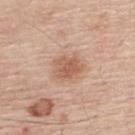Clinical impression: Imaged during a routine full-body skin examination; the lesion was not biopsied and no histopathology is available. Image and clinical context: The lesion-visualizer software estimated an area of roughly 7.5 mm², an eccentricity of roughly 0.6, and two-axis asymmetry of about 0.25. And it measured a within-lesion color-variation index near 2.5/10 and peripheral color asymmetry of about 0.5. Approximately 3.5 mm at its widest. A region of skin cropped from a whole-body photographic capture, roughly 15 mm wide. From the upper back. This is a white-light tile. A male patient, approximately 55 years of age.